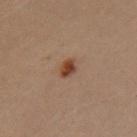Impression: Part of a total-body skin-imaging series; this lesion was reviewed on a skin check and was not flagged for biopsy. Background: On the left upper arm. Automated image analysis of the tile measured a footprint of about 3.5 mm², an outline eccentricity of about 0.8 (0 = round, 1 = elongated), and two-axis asymmetry of about 0.2. The analysis additionally found a classifier nevus-likeness of about 100/100 and lesion-presence confidence of about 100/100. A female subject aged around 30. A close-up tile cropped from a whole-body skin photograph, about 15 mm across.